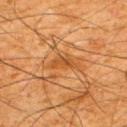Impression: Imaged during a routine full-body skin examination; the lesion was not biopsied and no histopathology is available. Background: Automated tile analysis of the lesion measured a lesion area of about 4.5 mm², an outline eccentricity of about 0.8 (0 = round, 1 = elongated), and two-axis asymmetry of about 0.45. And it measured a color-variation rating of about 2.5/10 and peripheral color asymmetry of about 1. The subject is a male in their mid- to late 60s. Located on the back. Cropped from a whole-body photographic skin survey; the tile spans about 15 mm.